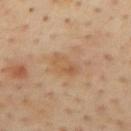Clinical impression: Captured during whole-body skin photography for melanoma surveillance; the lesion was not biopsied. Image and clinical context: The lesion is located on the mid back. The tile uses cross-polarized illumination. A 15 mm close-up tile from a total-body photography series done for melanoma screening. The subject is a male aged 53 to 57.